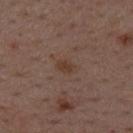biopsy status: total-body-photography surveillance lesion; no biopsy
anatomic site: the mid back
image source: ~15 mm crop, total-body skin-cancer survey
patient: male, roughly 50 years of age